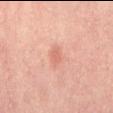A close-up tile cropped from a whole-body skin photograph, about 15 mm across. From the mid back. A male patient roughly 30 years of age.